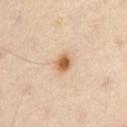Case summary:
– follow-up: catalogued during a skin exam; not biopsied
– image-analysis metrics: a lesion area of about 4 mm², an eccentricity of roughly 0.65, and a symmetry-axis asymmetry near 0.15; a mean CIELAB color near L≈62 a*≈21 b*≈38, a lesion–skin lightness drop of about 15, and a normalized border contrast of about 10; border irregularity of about 1.5 on a 0–10 scale, a color-variation rating of about 3/10, and peripheral color asymmetry of about 1; a nevus-likeness score of about 100/100 and lesion-presence confidence of about 100/100
– illumination: cross-polarized illumination
– location: the abdomen
– acquisition: ~15 mm crop, total-body skin-cancer survey
– subject: male, in their mid-50s